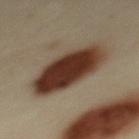workup: no biopsy performed (imaged during a skin exam)
lesion diameter: about 8 mm
anatomic site: the upper back
automated metrics: a footprint of about 28 mm², a shape eccentricity near 0.85, and two-axis asymmetry of about 0.1; an average lesion color of about L≈36 a*≈18 b*≈26 (CIELAB) and a lesion–skin lightness drop of about 19; a border-irregularity rating of about 1.5/10 and internal color variation of about 8 on a 0–10 scale
acquisition: ~15 mm tile from a whole-body skin photo
patient: female, aged 38 to 42
illumination: cross-polarized illumination